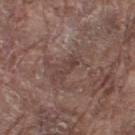Part of a total-body skin-imaging series; this lesion was reviewed on a skin check and was not flagged for biopsy. The patient is a male in their 80s. A 15 mm close-up tile from a total-body photography series done for melanoma screening. The lesion is on the right thigh.